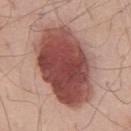Imaged during a routine full-body skin examination; the lesion was not biopsied and no histopathology is available. Cropped from a whole-body photographic skin survey; the tile spans about 15 mm. Automated tile analysis of the lesion measured two-axis asymmetry of about 0.1. The analysis additionally found an automated nevus-likeness rating near 100 out of 100 and a lesion-detection confidence of about 100/100. Longest diameter approximately 11 mm. The subject is a male approximately 70 years of age. The lesion is located on the chest.